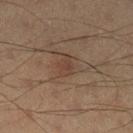On the left lower leg.
A male subject, roughly 40 years of age.
The recorded lesion diameter is about 2.5 mm.
The tile uses cross-polarized illumination.
A lesion tile, about 15 mm wide, cut from a 3D total-body photograph.